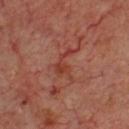Findings:
• notes — catalogued during a skin exam; not biopsied
• acquisition — ~15 mm tile from a whole-body skin photo
• diameter — ~4 mm (longest diameter)
• body site — the chest
• subject — male, aged 68 to 72
• lighting — cross-polarized illumination